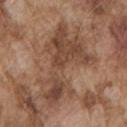biopsy_status: not biopsied; imaged during a skin examination
patient:
  sex: male
  age_approx: 75
lesion_size:
  long_diameter_mm_approx: 9.0
image:
  source: total-body photography crop
  field_of_view_mm: 15
automated_metrics:
  border_irregularity_0_10: 9.5
  color_variation_0_10: 4.5
  peripheral_color_asymmetry: 1.5
  nevus_likeness_0_100: 0
site: left upper arm